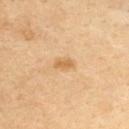Notes:
• notes · imaged on a skin check; not biopsied
• subject · female, aged 48–52
• image-analysis metrics · a lesion area of about 2.5 mm², an outline eccentricity of about 0.85 (0 = round, 1 = elongated), and two-axis asymmetry of about 0.2; a mean CIELAB color near L≈62 a*≈19 b*≈42, a lesion–skin lightness drop of about 9, and a lesion-to-skin contrast of about 7 (normalized; higher = more distinct); a border-irregularity index near 2/10; a classifier nevus-likeness of about 15/100 and a detector confidence of about 100 out of 100 that the crop contains a lesion
• acquisition · total-body-photography crop, ~15 mm field of view
• body site · the chest